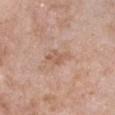Q: Is there a histopathology result?
A: catalogued during a skin exam; not biopsied
Q: What is the imaging modality?
A: total-body-photography crop, ~15 mm field of view
Q: Lesion location?
A: the front of the torso
Q: What are the patient's age and sex?
A: female, aged 73–77
Q: What is the lesion's diameter?
A: ≈3 mm
Q: How was the tile lit?
A: white-light
Q: What did automated image analysis measure?
A: an area of roughly 4 mm², an eccentricity of roughly 0.8, and two-axis asymmetry of about 0.4; a mean CIELAB color near L≈58 a*≈20 b*≈30, roughly 8 lightness units darker than nearby skin, and a lesion-to-skin contrast of about 5.5 (normalized; higher = more distinct); a border-irregularity index near 4/10, a within-lesion color-variation index near 2/10, and a peripheral color-asymmetry measure near 0.5; a classifier nevus-likeness of about 0/100 and lesion-presence confidence of about 100/100